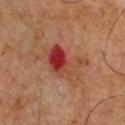biopsy status = imaged on a skin check; not biopsied
subject = male, aged approximately 50
anatomic site = the chest
image source = 15 mm crop, total-body photography